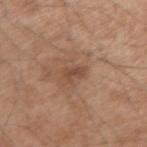biopsy status = no biopsy performed (imaged during a skin exam) | subject = male, in their mid- to late 50s | imaging modality = ~15 mm crop, total-body skin-cancer survey | anatomic site = the right upper arm.